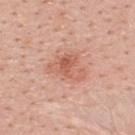Q: Was a biopsy performed?
A: catalogued during a skin exam; not biopsied
Q: What is the imaging modality?
A: ~15 mm tile from a whole-body skin photo
Q: Lesion location?
A: the upper back
Q: Lesion size?
A: ≈4.5 mm
Q: Who is the patient?
A: female, about 40 years old